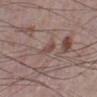Image and clinical context: A 15 mm crop from a total-body photograph taken for skin-cancer surveillance. Measured at roughly 3 mm in maximum diameter. From the left lower leg. A male patient aged approximately 75.